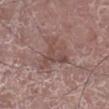Imaged during a routine full-body skin examination; the lesion was not biopsied and no histopathology is available. From the leg. This is a white-light tile. Measured at roughly 4 mm in maximum diameter. A male patient approximately 75 years of age. Cropped from a whole-body photographic skin survey; the tile spans about 15 mm. The total-body-photography lesion software estimated a lesion area of about 9 mm², an outline eccentricity of about 0.45 (0 = round, 1 = elongated), and a shape-asymmetry score of about 0.55 (0 = symmetric). It also reported a border-irregularity rating of about 6.5/10 and internal color variation of about 4 on a 0–10 scale.